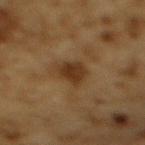Captured during whole-body skin photography for melanoma surveillance; the lesion was not biopsied.